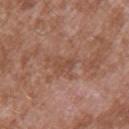Q: Was a biopsy performed?
A: catalogued during a skin exam; not biopsied
Q: Patient demographics?
A: male, aged around 45
Q: What is the imaging modality?
A: 15 mm crop, total-body photography
Q: How was the tile lit?
A: white-light
Q: Where on the body is the lesion?
A: the left upper arm
Q: How large is the lesion?
A: ≈3 mm
Q: Automated lesion metrics?
A: a footprint of about 4 mm², an eccentricity of roughly 0.8, and a shape-asymmetry score of about 0.3 (0 = symmetric); a mean CIELAB color near L≈48 a*≈21 b*≈29 and a normalized border contrast of about 5; a border-irregularity rating of about 3/10, internal color variation of about 2 on a 0–10 scale, and a peripheral color-asymmetry measure near 0.5